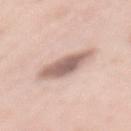biopsy status: catalogued during a skin exam; not biopsied | automated metrics: a lesion area of about 11 mm²; a lesion–skin lightness drop of about 15 and a normalized border contrast of about 9; a nevus-likeness score of about 20/100 and lesion-presence confidence of about 100/100 | image source: ~15 mm crop, total-body skin-cancer survey | subject: female, about 50 years old | body site: the mid back | size: about 5.5 mm.